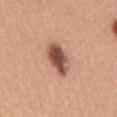Clinical impression:
Recorded during total-body skin imaging; not selected for excision or biopsy.
Image and clinical context:
The patient is a female aged 28–32. The lesion-visualizer software estimated an area of roughly 9 mm², an eccentricity of roughly 0.85, and two-axis asymmetry of about 0.2. It also reported a mean CIELAB color near L≈52 a*≈23 b*≈29, roughly 17 lightness units darker than nearby skin, and a normalized lesion–skin contrast near 11. And it measured a border-irregularity index near 2/10 and radial color variation of about 2. The analysis additionally found a classifier nevus-likeness of about 95/100 and a lesion-detection confidence of about 100/100. The tile uses white-light illumination. The lesion is located on the mid back. Cropped from a total-body skin-imaging series; the visible field is about 15 mm. The lesion's longest dimension is about 4.5 mm.